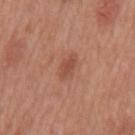The lesion was photographed on a routine skin check and not biopsied; there is no pathology result. The tile uses white-light illumination. From the mid back. A male patient aged approximately 70. Cropped from a total-body skin-imaging series; the visible field is about 15 mm. Measured at roughly 3.5 mm in maximum diameter. The lesion-visualizer software estimated an area of roughly 4.5 mm² and two-axis asymmetry of about 0.3. And it measured a mean CIELAB color near L≈50 a*≈25 b*≈30 and a normalized border contrast of about 6. The analysis additionally found a border-irregularity index near 3/10 and radial color variation of about 0.5.